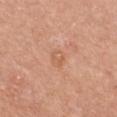Longest diameter approximately 2.5 mm. Imaged with white-light lighting. The subject is a female in their 60s. A roughly 15 mm field-of-view crop from a total-body skin photograph. The lesion is on the chest. Automated tile analysis of the lesion measured a mean CIELAB color near L≈61 a*≈23 b*≈35 and a normalized border contrast of about 4.5. The analysis additionally found a detector confidence of about 100 out of 100 that the crop contains a lesion.